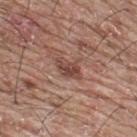Clinical impression: The lesion was tiled from a total-body skin photograph and was not biopsied. Acquisition and patient details: A roughly 15 mm field-of-view crop from a total-body skin photograph. Located on the upper back. A male subject in their mid- to late 60s.